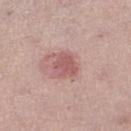Q: How was the tile lit?
A: white-light illumination
Q: What kind of image is this?
A: ~15 mm tile from a whole-body skin photo
Q: Who is the patient?
A: female, aged approximately 40
Q: Lesion size?
A: ~3 mm (longest diameter)
Q: What is the anatomic site?
A: the left thigh